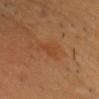<lesion>
<biopsy_status>not biopsied; imaged during a skin examination</biopsy_status>
<site>head or neck</site>
<image>
  <source>total-body photography crop</source>
  <field_of_view_mm>15</field_of_view_mm>
</image>
<patient>
  <sex>male</sex>
  <age_approx>60</age_approx>
</patient>
<lighting>cross-polarized</lighting>
</lesion>